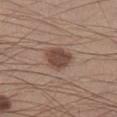No biopsy was performed on this lesion — it was imaged during a full skin examination and was not determined to be concerning. The recorded lesion diameter is about 3.5 mm. A 15 mm close-up tile from a total-body photography series done for melanoma screening. An algorithmic analysis of the crop reported a lesion area of about 8.5 mm² and two-axis asymmetry of about 0.25. The software also gave peripheral color asymmetry of about 1. A male subject, aged 28 to 32. The lesion is located on the left lower leg.